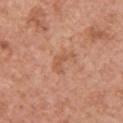Imaged during a routine full-body skin examination; the lesion was not biopsied and no histopathology is available.
The lesion is on the upper back.
The lesion-visualizer software estimated a shape eccentricity near 0.8 and two-axis asymmetry of about 0.55. The analysis additionally found an average lesion color of about L≈56 a*≈24 b*≈34 (CIELAB) and roughly 7 lightness units darker than nearby skin. And it measured a border-irregularity index near 6/10 and a peripheral color-asymmetry measure near 0. The software also gave an automated nevus-likeness rating near 0 out of 100 and lesion-presence confidence of about 100/100.
A male subject aged approximately 80.
A 15 mm close-up tile from a total-body photography series done for melanoma screening.
Captured under white-light illumination.